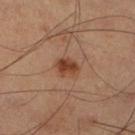<case>
<biopsy_status>not biopsied; imaged during a skin examination</biopsy_status>
<site>right lower leg</site>
<patient>
  <sex>male</sex>
  <age_approx>60</age_approx>
</patient>
<image>
  <source>total-body photography crop</source>
  <field_of_view_mm>15</field_of_view_mm>
</image>
<lesion_size>
  <long_diameter_mm_approx>3.0</long_diameter_mm_approx>
</lesion_size>
</case>